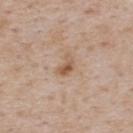site — the upper back | illumination — white-light | subject — male, in their mid-70s | acquisition — total-body-photography crop, ~15 mm field of view | diameter — ≈2.5 mm | automated lesion analysis — a footprint of about 3.5 mm², an eccentricity of roughly 0.8, and a symmetry-axis asymmetry near 0.35.